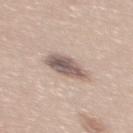Assessment: Part of a total-body skin-imaging series; this lesion was reviewed on a skin check and was not flagged for biopsy. Clinical summary: The patient is a female in their mid-30s. The recorded lesion diameter is about 4.5 mm. Captured under white-light illumination. A 15 mm close-up tile from a total-body photography series done for melanoma screening. Automated image analysis of the tile measured a shape eccentricity near 0.8. It also reported a border-irregularity rating of about 2/10 and a color-variation rating of about 5.5/10. It also reported an automated nevus-likeness rating near 30 out of 100 and a lesion-detection confidence of about 100/100. From the upper back.